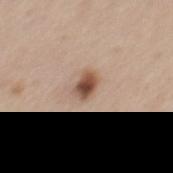patient:
  sex: male
  age_approx: 45
image:
  source: total-body photography crop
  field_of_view_mm: 15
lesion_size:
  long_diameter_mm_approx: 2.5
site: mid back
automated_metrics:
  area_mm2_approx: 4.5
  eccentricity: 0.7
  shape_asymmetry: 0.15
  cielab_L: 51
  cielab_a: 19
  cielab_b: 28
  vs_skin_darker_L: 15.0
  vs_skin_contrast_norm: 10.5
  border_irregularity_0_10: 1.5
  color_variation_0_10: 6.0
  peripheral_color_asymmetry: 1.5
  nevus_likeness_0_100: 95
  lesion_detection_confidence_0_100: 100